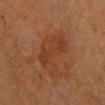follow-up: total-body-photography surveillance lesion; no biopsy | subject: female, about 55 years old | body site: the right forearm | lighting: cross-polarized illumination | image: 15 mm crop, total-body photography.